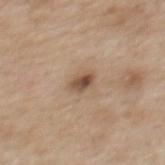Q: Was a biopsy performed?
A: total-body-photography surveillance lesion; no biopsy
Q: Who is the patient?
A: female, aged 38 to 42
Q: Lesion size?
A: about 2.5 mm
Q: What kind of image is this?
A: 15 mm crop, total-body photography
Q: Lesion location?
A: the mid back